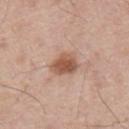This lesion was catalogued during total-body skin photography and was not selected for biopsy.
A close-up tile cropped from a whole-body skin photograph, about 15 mm across.
Imaged with white-light lighting.
The lesion's longest dimension is about 3.5 mm.
The lesion is on the mid back.
A male patient, aged around 65.